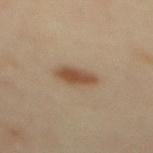Assessment:
The lesion was tiled from a total-body skin photograph and was not biopsied.
Acquisition and patient details:
A lesion tile, about 15 mm wide, cut from a 3D total-body photograph. The recorded lesion diameter is about 4 mm. The patient is a female approximately 40 years of age. Imaged with cross-polarized lighting. Located on the mid back.